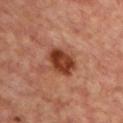Impression: Captured during whole-body skin photography for melanoma surveillance; the lesion was not biopsied. Acquisition and patient details: A female subject aged around 45. A lesion tile, about 15 mm wide, cut from a 3D total-body photograph. Imaged with cross-polarized lighting. From the chest.